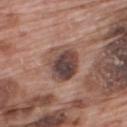biopsy_status: not biopsied; imaged during a skin examination
image:
  source: total-body photography crop
  field_of_view_mm: 15
site: upper back
patient:
  sex: male
  age_approx: 70